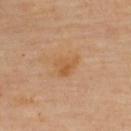Case summary:
• follow-up · imaged on a skin check; not biopsied
• lesion diameter · ≈3 mm
• image · total-body-photography crop, ~15 mm field of view
• automated metrics · an area of roughly 5.5 mm² and an outline eccentricity of about 0.7 (0 = round, 1 = elongated); an average lesion color of about L≈57 a*≈21 b*≈40 (CIELAB) and roughly 7 lightness units darker than nearby skin
• anatomic site · the back
• subject · female, about 60 years old